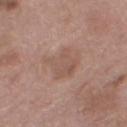Background:
On the left thigh. The patient is a female aged around 70. This image is a 15 mm lesion crop taken from a total-body photograph.